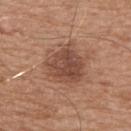Findings:
– workup · total-body-photography surveillance lesion; no biopsy
– TBP lesion metrics · a lesion color around L≈47 a*≈21 b*≈28 in CIELAB and a lesion–skin lightness drop of about 10; a border-irregularity index near 2.5/10, a color-variation rating of about 4/10, and a peripheral color-asymmetry measure near 1.5; a nevus-likeness score of about 55/100
– patient · male, aged approximately 65
– lighting · white-light illumination
– acquisition · ~15 mm tile from a whole-body skin photo
– location · the upper back